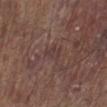Impression:
This lesion was catalogued during total-body skin photography and was not selected for biopsy.
Context:
A male subject approximately 80 years of age. Imaged with white-light lighting. A lesion tile, about 15 mm wide, cut from a 3D total-body photograph. From the leg. The recorded lesion diameter is about 2.5 mm.